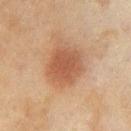This lesion was catalogued during total-body skin photography and was not selected for biopsy.
From the left thigh.
A close-up tile cropped from a whole-body skin photograph, about 15 mm across.
A female subject, aged 58–62.